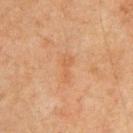biopsy status = total-body-photography surveillance lesion; no biopsy
subject = male, about 65 years old
imaging modality = total-body-photography crop, ~15 mm field of view
site = the chest
TBP lesion metrics = an area of roughly 3.5 mm² and a symmetry-axis asymmetry near 0.4; an average lesion color of about L≈51 a*≈21 b*≈35 (CIELAB) and roughly 5 lightness units darker than nearby skin